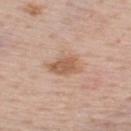subject — male, in their 60s
body site — the upper back
automated lesion analysis — an outline eccentricity of about 0.7 (0 = round, 1 = elongated) and a shape-asymmetry score of about 0.3 (0 = symmetric)
illumination — white-light illumination
image source — 15 mm crop, total-body photography